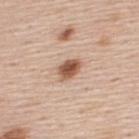No biopsy was performed on this lesion — it was imaged during a full skin examination and was not determined to be concerning.
This is a white-light tile.
A 15 mm close-up extracted from a 3D total-body photography capture.
Located on the upper back.
A male subject in their mid- to late 40s.
The lesion's longest dimension is about 3 mm.